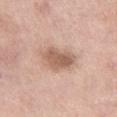{
  "biopsy_status": "not biopsied; imaged during a skin examination",
  "patient": {
    "sex": "female",
    "age_approx": 55
  },
  "site": "right thigh",
  "image": {
    "source": "total-body photography crop",
    "field_of_view_mm": 15
  },
  "lesion_size": {
    "long_diameter_mm_approx": 4.0
  }
}